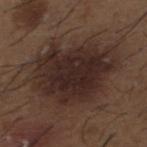Q: Was this lesion biopsied?
A: total-body-photography surveillance lesion; no biopsy
Q: What are the patient's age and sex?
A: male, aged approximately 50
Q: Where on the body is the lesion?
A: the upper back
Q: How was the tile lit?
A: white-light
Q: What is the lesion's diameter?
A: about 10 mm
Q: What kind of image is this?
A: total-body-photography crop, ~15 mm field of view
Q: What did automated image analysis measure?
A: an outline eccentricity of about 0.75 (0 = round, 1 = elongated) and a shape-asymmetry score of about 0.2 (0 = symmetric); a mean CIELAB color near L≈27 a*≈15 b*≈19, a lesion–skin lightness drop of about 10, and a normalized lesion–skin contrast near 11; an automated nevus-likeness rating near 35 out of 100 and a lesion-detection confidence of about 100/100